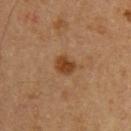This lesion was catalogued during total-body skin photography and was not selected for biopsy. Cropped from a whole-body photographic skin survey; the tile spans about 15 mm. The patient is a male in their 60s.